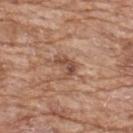Captured during whole-body skin photography for melanoma surveillance; the lesion was not biopsied. A male subject aged 58–62. Captured under white-light illumination. A 15 mm close-up extracted from a 3D total-body photography capture. The recorded lesion diameter is about 3 mm. The lesion is on the chest.